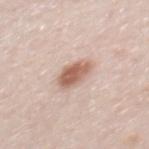<case>
<biopsy_status>not biopsied; imaged during a skin examination</biopsy_status>
<automated_metrics>
  <border_irregularity_0_10>1.5</border_irregularity_0_10>
  <color_variation_0_10>4.0</color_variation_0_10>
  <peripheral_color_asymmetry>1.5</peripheral_color_asymmetry>
</automated_metrics>
<image>
  <source>total-body photography crop</source>
  <field_of_view_mm>15</field_of_view_mm>
</image>
<patient>
  <sex>male</sex>
  <age_approx>40</age_approx>
</patient>
<site>mid back</site>
<lighting>white-light</lighting>
<lesion_size>
  <long_diameter_mm_approx>4.0</long_diameter_mm_approx>
</lesion_size>
</case>